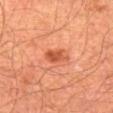biopsy_status: not biopsied; imaged during a skin examination
image:
  source: total-body photography crop
  field_of_view_mm: 15
lighting: cross-polarized
patient:
  sex: male
  age_approx: 65
site: left thigh
lesion_size:
  long_diameter_mm_approx: 3.5